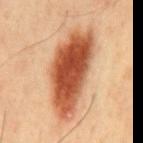Impression: Recorded during total-body skin imaging; not selected for excision or biopsy. Background: A 15 mm close-up extracted from a 3D total-body photography capture. Imaged with cross-polarized lighting. Measured at roughly 10.5 mm in maximum diameter. Located on the mid back. A male patient in their mid-40s.